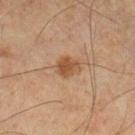This lesion was catalogued during total-body skin photography and was not selected for biopsy.
Approximately 3 mm at its widest.
On the left lower leg.
The tile uses cross-polarized illumination.
An algorithmic analysis of the crop reported a footprint of about 5 mm², an outline eccentricity of about 0.6 (0 = round, 1 = elongated), and a shape-asymmetry score of about 0.25 (0 = symmetric). The software also gave an average lesion color of about L≈45 a*≈19 b*≈32 (CIELAB), about 9 CIELAB-L* units darker than the surrounding skin, and a lesion-to-skin contrast of about 8 (normalized; higher = more distinct). And it measured lesion-presence confidence of about 100/100.
A close-up tile cropped from a whole-body skin photograph, about 15 mm across.
The subject is a male aged approximately 60.